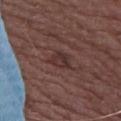{
  "biopsy_status": "not biopsied; imaged during a skin examination",
  "site": "upper back",
  "image": {
    "source": "total-body photography crop",
    "field_of_view_mm": 15
  },
  "patient": {
    "sex": "female",
    "age_approx": 75
  },
  "lighting": "white-light",
  "lesion_size": {
    "long_diameter_mm_approx": 3.0
  }
}